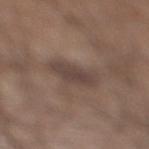Q: Is there a histopathology result?
A: catalogued during a skin exam; not biopsied
Q: Automated lesion metrics?
A: an outline eccentricity of about 0.9 (0 = round, 1 = elongated) and a shape-asymmetry score of about 0.25 (0 = symmetric)
Q: What kind of image is this?
A: 15 mm crop, total-body photography
Q: What are the patient's age and sex?
A: male, approximately 55 years of age
Q: What is the anatomic site?
A: the right lower leg
Q: What lighting was used for the tile?
A: white-light illumination